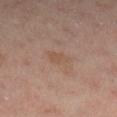{"biopsy_status": "not biopsied; imaged during a skin examination", "image": {"source": "total-body photography crop", "field_of_view_mm": 15}, "patient": {"sex": "female", "age_approx": 40}, "site": "leg"}